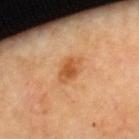| field | value |
|---|---|
| automated metrics | a lesion area of about 6 mm² and a symmetry-axis asymmetry near 0.25; a lesion–skin lightness drop of about 10 and a normalized border contrast of about 7.5; a classifier nevus-likeness of about 85/100 and a detector confidence of about 100 out of 100 that the crop contains a lesion |
| lesion size | ~3 mm (longest diameter) |
| subject | female, approximately 70 years of age |
| image | 15 mm crop, total-body photography |
| site | the upper back |
| illumination | cross-polarized illumination |